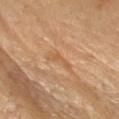Notes:
• workup — imaged on a skin check; not biopsied
• site — the right forearm
• illumination — cross-polarized
• image source — 15 mm crop, total-body photography
• diameter — about 3 mm
• patient — female, roughly 70 years of age
• TBP lesion metrics — a lesion area of about 3.5 mm² and two-axis asymmetry of about 0.5; a lesion color around L≈59 a*≈22 b*≈39 in CIELAB, about 6 CIELAB-L* units darker than the surrounding skin, and a normalized border contrast of about 5; a classifier nevus-likeness of about 0/100 and a lesion-detection confidence of about 100/100